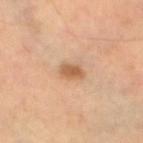Assessment: The lesion was tiled from a total-body skin photograph and was not biopsied. Image and clinical context: The lesion is located on the right leg. Approximately 2.5 mm at its widest. The tile uses cross-polarized illumination. The total-body-photography lesion software estimated a border-irregularity index near 2.5/10. A close-up tile cropped from a whole-body skin photograph, about 15 mm across. The subject is a female aged 58 to 62.